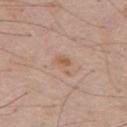<record>
  <biopsy_status>not biopsied; imaged during a skin examination</biopsy_status>
  <lighting>white-light</lighting>
  <lesion_size>
    <long_diameter_mm_approx>3.0</long_diameter_mm_approx>
  </lesion_size>
  <image>
    <source>total-body photography crop</source>
    <field_of_view_mm>15</field_of_view_mm>
  </image>
  <automated_metrics>
    <cielab_L>58</cielab_L>
    <cielab_a>19</cielab_a>
    <cielab_b>31</cielab_b>
    <vs_skin_darker_L>7.0</vs_skin_darker_L>
    <vs_skin_contrast_norm>6.5</vs_skin_contrast_norm>
    <border_irregularity_0_10>4.0</border_irregularity_0_10>
    <peripheral_color_asymmetry>1.0</peripheral_color_asymmetry>
    <nevus_likeness_0_100>0</nevus_likeness_0_100>
    <lesion_detection_confidence_0_100>100</lesion_detection_confidence_0_100>
  </automated_metrics>
  <patient>
    <sex>male</sex>
    <age_approx>60</age_approx>
  </patient>
  <site>chest</site>
</record>